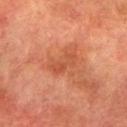| key | value |
|---|---|
| notes | total-body-photography surveillance lesion; no biopsy |
| TBP lesion metrics | a footprint of about 4.5 mm², a shape eccentricity near 0.95, and a shape-asymmetry score of about 0.35 (0 = symmetric); a border-irregularity index near 4.5/10, internal color variation of about 1.5 on a 0–10 scale, and a peripheral color-asymmetry measure near 0.5 |
| imaging modality | 15 mm crop, total-body photography |
| subject | male, aged 73 to 77 |
| lighting | cross-polarized illumination |
| diameter | about 3.5 mm |
| location | the left lower leg |